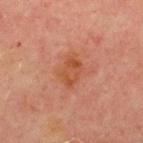| feature | finding |
|---|---|
| follow-up | total-body-photography surveillance lesion; no biopsy |
| image-analysis metrics | an outline eccentricity of about 0.8 (0 = round, 1 = elongated) and a shape-asymmetry score of about 0.25 (0 = symmetric); a mean CIELAB color near L≈41 a*≈24 b*≈31, about 6 CIELAB-L* units darker than the surrounding skin, and a normalized lesion–skin contrast near 7 |
| subject | male, aged 68–72 |
| imaging modality | ~15 mm crop, total-body skin-cancer survey |
| body site | the chest |
| illumination | cross-polarized illumination |
| diameter | ~3.5 mm (longest diameter) |